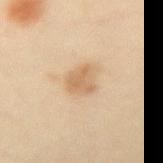A roughly 15 mm field-of-view crop from a total-body skin photograph.
This is a cross-polarized tile.
Located on the right forearm.
Measured at roughly 3 mm in maximum diameter.
The lesion-visualizer software estimated border irregularity of about 4 on a 0–10 scale, a color-variation rating of about 1.5/10, and radial color variation of about 0.5.
A female patient in their 20s.